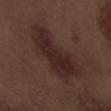{"biopsy_status": "not biopsied; imaged during a skin examination", "lesion_size": {"long_diameter_mm_approx": 8.5}, "image": {"source": "total-body photography crop", "field_of_view_mm": 15}, "patient": {"sex": "male", "age_approx": 70}, "lighting": "white-light", "site": "left forearm"}